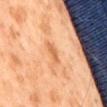| feature | finding |
|---|---|
| follow-up | imaged on a skin check; not biopsied |
| image | 15 mm crop, total-body photography |
| subject | male, roughly 60 years of age |
| lighting | cross-polarized illumination |
| TBP lesion metrics | a footprint of about 3 mm², an outline eccentricity of about 0.85 (0 = round, 1 = elongated), and a shape-asymmetry score of about 0.35 (0 = symmetric); a normalized lesion–skin contrast near 6.5; a border-irregularity rating of about 3.5/10, a within-lesion color-variation index near 0/10, and peripheral color asymmetry of about 0 |
| size | ≈2.5 mm |
| anatomic site | the abdomen |